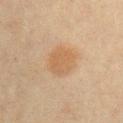Q: Automated lesion metrics?
A: an average lesion color of about L≈49 a*≈16 b*≈31 (CIELAB) and a normalized border contrast of about 5.5; a nevus-likeness score of about 55/100 and a detector confidence of about 100 out of 100 that the crop contains a lesion
Q: What kind of image is this?
A: total-body-photography crop, ~15 mm field of view
Q: What is the anatomic site?
A: the right upper arm
Q: Illumination type?
A: cross-polarized illumination
Q: Who is the patient?
A: male, aged 58–62